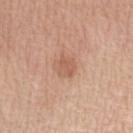Assessment: The lesion was tiled from a total-body skin photograph and was not biopsied. Background: Longest diameter approximately 2.5 mm. A female subject, aged around 55. On the arm. A lesion tile, about 15 mm wide, cut from a 3D total-body photograph. This is a white-light tile.